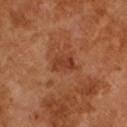Captured during whole-body skin photography for melanoma surveillance; the lesion was not biopsied. Longest diameter approximately 3 mm. A male patient aged 63–67. A 15 mm close-up tile from a total-body photography series done for melanoma screening.